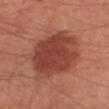follow-up: imaged on a skin check; not biopsied
image source: total-body-photography crop, ~15 mm field of view
body site: the right thigh
patient: male, aged 63 to 67